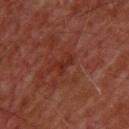No biopsy was performed on this lesion — it was imaged during a full skin examination and was not determined to be concerning.
Approximately 2.5 mm at its widest.
On the head or neck.
A 15 mm crop from a total-body photograph taken for skin-cancer surveillance.
The subject is a male approximately 60 years of age.
The tile uses cross-polarized illumination.